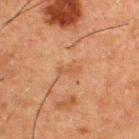Impression:
The lesion was tiled from a total-body skin photograph and was not biopsied.
Context:
The patient is a male aged approximately 50. The lesion is located on the upper back. Measured at roughly 2.5 mm in maximum diameter. An algorithmic analysis of the crop reported an area of roughly 2.5 mm², an eccentricity of roughly 0.95, and a shape-asymmetry score of about 0.3 (0 = symmetric). The software also gave a mean CIELAB color near L≈43 a*≈20 b*≈31, a lesion–skin lightness drop of about 5, and a normalized lesion–skin contrast near 5. It also reported an automated nevus-likeness rating near 0 out of 100 and a detector confidence of about 100 out of 100 that the crop contains a lesion. Captured under cross-polarized illumination. A lesion tile, about 15 mm wide, cut from a 3D total-body photograph.